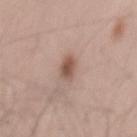Q: Lesion location?
A: the back
Q: How was this image acquired?
A: 15 mm crop, total-body photography
Q: Illumination type?
A: white-light
Q: What are the patient's age and sex?
A: male, about 40 years old
Q: Automated lesion metrics?
A: a lesion color around L≈53 a*≈20 b*≈25 in CIELAB, a lesion–skin lightness drop of about 12, and a lesion-to-skin contrast of about 8.5 (normalized; higher = more distinct); a border-irregularity rating of about 2.5/10 and internal color variation of about 2 on a 0–10 scale